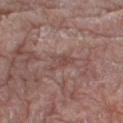The lesion was photographed on a routine skin check and not biopsied; there is no pathology result.
Imaged with white-light lighting.
A male subject, roughly 80 years of age.
A close-up tile cropped from a whole-body skin photograph, about 15 mm across.
Located on the leg.
Measured at roughly 3.5 mm in maximum diameter.